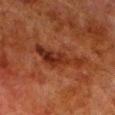{
  "biopsy_status": "not biopsied; imaged during a skin examination",
  "image": {
    "source": "total-body photography crop",
    "field_of_view_mm": 15
  },
  "patient": {
    "sex": "male",
    "age_approx": 80
  },
  "lighting": "cross-polarized",
  "site": "left lower leg",
  "lesion_size": {
    "long_diameter_mm_approx": 7.0
  },
  "automated_metrics": {
    "area_mm2_approx": 11.0,
    "eccentricity": 0.95,
    "shape_asymmetry": 0.55,
    "color_variation_0_10": 4.5,
    "peripheral_color_asymmetry": 1.0
  }
}